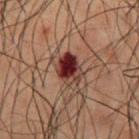- anatomic site — the abdomen
- illumination — cross-polarized illumination
- image — ~15 mm tile from a whole-body skin photo
- patient — male, approximately 50 years of age
- lesion size — ≈3.5 mm